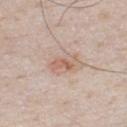Assessment: Recorded during total-body skin imaging; not selected for excision or biopsy. Background: The tile uses white-light illumination. The lesion is on the chest. A 15 mm crop from a total-body photograph taken for skin-cancer surveillance. Longest diameter approximately 3.5 mm. The patient is a male about 50 years old. The total-body-photography lesion software estimated an outline eccentricity of about 0.85 (0 = round, 1 = elongated) and two-axis asymmetry of about 0.35. The analysis additionally found border irregularity of about 4.5 on a 0–10 scale, a within-lesion color-variation index near 4.5/10, and radial color variation of about 1.5.